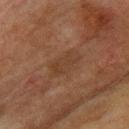A male patient in their mid-70s.
Measured at roughly 4.5 mm in maximum diameter.
The lesion is located on the back.
Cropped from a total-body skin-imaging series; the visible field is about 15 mm.
The lesion-visualizer software estimated a shape eccentricity near 0.85 and a symmetry-axis asymmetry near 0.3. The analysis additionally found an average lesion color of about L≈32 a*≈16 b*≈25 (CIELAB) and a lesion-to-skin contrast of about 5 (normalized; higher = more distinct). And it measured a classifier nevus-likeness of about 0/100.
The tile uses cross-polarized illumination.